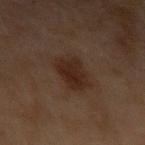Part of a total-body skin-imaging series; this lesion was reviewed on a skin check and was not flagged for biopsy. Approximately 3.5 mm at its widest. From the arm. A lesion tile, about 15 mm wide, cut from a 3D total-body photograph. The tile uses cross-polarized illumination. A male subject, aged 68 to 72. The lesion-visualizer software estimated a lesion area of about 10 mm² and two-axis asymmetry of about 0.15. The software also gave a mean CIELAB color near L≈18 a*≈13 b*≈18, about 6 CIELAB-L* units darker than the surrounding skin, and a normalized lesion–skin contrast near 8.5. The analysis additionally found a classifier nevus-likeness of about 85/100.